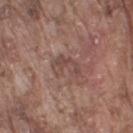notes = imaged on a skin check; not biopsied
lighting = white-light illumination
automated lesion analysis = a footprint of about 5.5 mm², a shape eccentricity near 0.8, and a symmetry-axis asymmetry near 0.6; an automated nevus-likeness rating near 0 out of 100 and a lesion-detection confidence of about 95/100
patient = male, aged 73 to 77
image source = total-body-photography crop, ~15 mm field of view
body site = the arm
lesion size = ≈3.5 mm